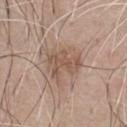Part of a total-body skin-imaging series; this lesion was reviewed on a skin check and was not flagged for biopsy. The patient is a male aged 63–67. A region of skin cropped from a whole-body photographic capture, roughly 15 mm wide. Located on the chest. Longest diameter approximately 4 mm. This is a white-light tile.